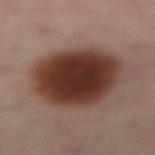The lesion was tiled from a total-body skin photograph and was not biopsied.
Captured under cross-polarized illumination.
The subject is a male aged approximately 40.
A 15 mm close-up tile from a total-body photography series done for melanoma screening.
The recorded lesion diameter is about 7.5 mm.
From the back.